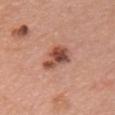<case>
<biopsy_status>not biopsied; imaged during a skin examination</biopsy_status>
<image>
  <source>total-body photography crop</source>
  <field_of_view_mm>15</field_of_view_mm>
</image>
<lesion_size>
  <long_diameter_mm_approx>4.0</long_diameter_mm_approx>
</lesion_size>
<site>front of the torso</site>
<automated_metrics>
  <area_mm2_approx>8.5</area_mm2_approx>
  <eccentricity>0.7</eccentricity>
  <shape_asymmetry>0.35</shape_asymmetry>
</automated_metrics>
<patient>
  <sex>male</sex>
  <age_approx>60</age_approx>
</patient>
<lighting>white-light</lighting>
</case>